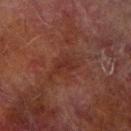Q: Was this lesion biopsied?
A: no biopsy performed (imaged during a skin exam)
Q: Illumination type?
A: cross-polarized illumination
Q: What kind of image is this?
A: 15 mm crop, total-body photography
Q: Lesion size?
A: ≈3 mm
Q: What is the anatomic site?
A: the right forearm
Q: Who is the patient?
A: male, aged 73 to 77
Q: Automated lesion metrics?
A: an area of roughly 4.5 mm², an eccentricity of roughly 0.8, and a shape-asymmetry score of about 0.3 (0 = symmetric); a lesion color around L≈25 a*≈21 b*≈22 in CIELAB; a border-irregularity index near 3/10, a within-lesion color-variation index near 2/10, and a peripheral color-asymmetry measure near 1; a nevus-likeness score of about 0/100